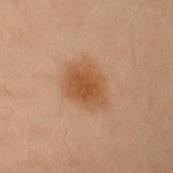biopsy status: imaged on a skin check; not biopsied | location: the left arm | lighting: cross-polarized illumination | patient: female, aged 53 to 57 | size: ~5.5 mm (longest diameter) | imaging modality: 15 mm crop, total-body photography | TBP lesion metrics: an outline eccentricity of about 0.7 (0 = round, 1 = elongated) and a shape-asymmetry score of about 0.2 (0 = symmetric); a mean CIELAB color near L≈44 a*≈18 b*≈30 and roughly 8 lightness units darker than nearby skin; internal color variation of about 3 on a 0–10 scale and peripheral color asymmetry of about 0.5.